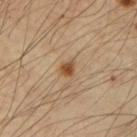Part of a total-body skin-imaging series; this lesion was reviewed on a skin check and was not flagged for biopsy.
The lesion's longest dimension is about 2 mm.
The patient is a male in their mid-60s.
Captured under cross-polarized illumination.
Automated tile analysis of the lesion measured about 12 CIELAB-L* units darker than the surrounding skin and a normalized border contrast of about 9.
Located on the right upper arm.
Cropped from a whole-body photographic skin survey; the tile spans about 15 mm.